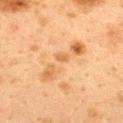The total-body-photography lesion software estimated a lesion color around L≈56 a*≈19 b*≈37 in CIELAB and a normalized lesion–skin contrast near 5. It also reported an automated nevus-likeness rating near 0 out of 100 and a detector confidence of about 100 out of 100 that the crop contains a lesion. This is a cross-polarized tile. A lesion tile, about 15 mm wide, cut from a 3D total-body photograph. The recorded lesion diameter is about 7 mm. The patient is a female about 40 years old. The lesion is located on the upper back.